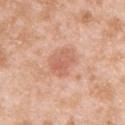The lesion was photographed on a routine skin check and not biopsied; there is no pathology result. A 15 mm close-up extracted from a 3D total-body photography capture. A male subject, about 25 years old. The lesion is located on the right upper arm.